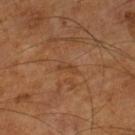A male patient, aged 63 to 67. Approximately 2.5 mm at its widest. A 15 mm crop from a total-body photograph taken for skin-cancer surveillance. The tile uses cross-polarized illumination. Located on the leg. Automated tile analysis of the lesion measured a footprint of about 2 mm² and an eccentricity of roughly 0.9. It also reported about 5 CIELAB-L* units darker than the surrounding skin and a normalized border contrast of about 5.